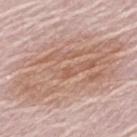lesion_size:
  long_diameter_mm_approx: 14.0
site: upper back
image:
  source: total-body photography crop
  field_of_view_mm: 15
lighting: white-light
automated_metrics:
  shape_asymmetry: 0.25
  cielab_L: 62
  cielab_a: 18
  cielab_b: 27
  vs_skin_darker_L: 9.0
  border_irregularity_0_10: 6.0
  color_variation_0_10: 5.5
  peripheral_color_asymmetry: 2.0
  nevus_likeness_0_100: 25
  lesion_detection_confidence_0_100: 100
patient:
  sex: male
  age_approx: 65